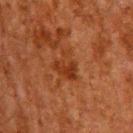{"biopsy_status": "not biopsied; imaged during a skin examination", "image": {"source": "total-body photography crop", "field_of_view_mm": 15}, "site": "back", "patient": {"sex": "male", "age_approx": 60}}